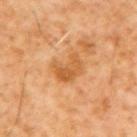Captured during whole-body skin photography for melanoma surveillance; the lesion was not biopsied.
Approximately 4 mm at its widest.
From the upper back.
A 15 mm close-up extracted from a 3D total-body photography capture.
A male patient, aged approximately 60.